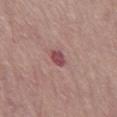image source — 15 mm crop, total-body photography
lighting — white-light
location — the right thigh
patient — female, aged 63 to 67